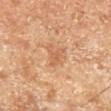Case summary:
– acquisition · total-body-photography crop, ~15 mm field of view
– automated lesion analysis · a lesion color around L≈59 a*≈22 b*≈35 in CIELAB, about 8 CIELAB-L* units darker than the surrounding skin, and a normalized lesion–skin contrast near 5
– anatomic site · the right lower leg
– lighting · cross-polarized illumination
– subject · male, aged approximately 70